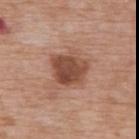{"biopsy_status": "not biopsied; imaged during a skin examination", "patient": {"sex": "female", "age_approx": 75}, "site": "back", "lighting": "white-light", "image": {"source": "total-body photography crop", "field_of_view_mm": 15}, "lesion_size": {"long_diameter_mm_approx": 4.0}}